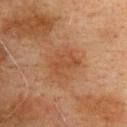Clinical impression:
Imaged during a routine full-body skin examination; the lesion was not biopsied and no histopathology is available.
Context:
Located on the upper back. A close-up tile cropped from a whole-body skin photograph, about 15 mm across. The patient is a male in their mid- to late 70s. This is a cross-polarized tile. Automated tile analysis of the lesion measured a footprint of about 8.5 mm², a shape eccentricity near 0.7, and a shape-asymmetry score of about 0.25 (0 = symmetric).